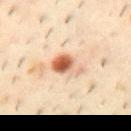Impression:
Recorded during total-body skin imaging; not selected for excision or biopsy.
Context:
A male subject aged 38–42. On the mid back. The tile uses cross-polarized illumination. Approximately 3.5 mm at its widest. A region of skin cropped from a whole-body photographic capture, roughly 15 mm wide.